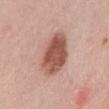Q: Was a biopsy performed?
A: no biopsy performed (imaged during a skin exam)
Q: Where on the body is the lesion?
A: the mid back
Q: Who is the patient?
A: male, approximately 50 years of age
Q: What did automated image analysis measure?
A: a lesion area of about 17 mm², a shape eccentricity near 0.65, and a symmetry-axis asymmetry near 0.15; a classifier nevus-likeness of about 100/100
Q: What is the lesion's diameter?
A: ~5.5 mm (longest diameter)
Q: How was this image acquired?
A: ~15 mm tile from a whole-body skin photo